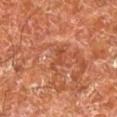Impression:
Recorded during total-body skin imaging; not selected for excision or biopsy.
Image and clinical context:
On the left lower leg. Cropped from a total-body skin-imaging series; the visible field is about 15 mm. About 2.5 mm across. A male patient in their mid-60s.